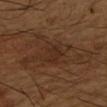Recorded during total-body skin imaging; not selected for excision or biopsy. From the right forearm. A male subject, approximately 65 years of age. A region of skin cropped from a whole-body photographic capture, roughly 15 mm wide. The lesion's longest dimension is about 9 mm.